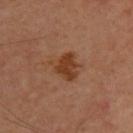Cropped from a whole-body photographic skin survey; the tile spans about 15 mm. On the upper back. Captured under cross-polarized illumination. The lesion's longest dimension is about 4 mm. A male patient, roughly 60 years of age.